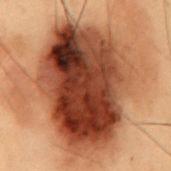| key | value |
|---|---|
| biopsy status | no biopsy performed (imaged during a skin exam) |
| anatomic site | the mid back |
| automated metrics | a mean CIELAB color near L≈34 a*≈23 b*≈28, a lesion–skin lightness drop of about 20, and a normalized border contrast of about 15.5; a detector confidence of about 100 out of 100 that the crop contains a lesion |
| patient | male, approximately 55 years of age |
| diameter | about 12 mm |
| image source | total-body-photography crop, ~15 mm field of view |
| illumination | cross-polarized |